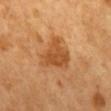Impression: Recorded during total-body skin imaging; not selected for excision or biopsy. Background: A male patient roughly 65 years of age. Cropped from a total-body skin-imaging series; the visible field is about 15 mm. The lesion is on the back.